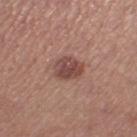biopsy status — catalogued during a skin exam; not biopsied | anatomic site — the leg | imaging modality — total-body-photography crop, ~15 mm field of view | size — ≈3 mm | patient — female, about 40 years old.